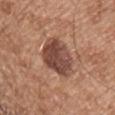<case>
<image>
  <source>total-body photography crop</source>
  <field_of_view_mm>15</field_of_view_mm>
</image>
<patient>
  <sex>male</sex>
  <age_approx>55</age_approx>
</patient>
<lighting>white-light</lighting>
<lesion_size>
  <long_diameter_mm_approx>4.5</long_diameter_mm_approx>
</lesion_size>
<site>left upper arm</site>
</case>